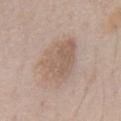This lesion was catalogued during total-body skin photography and was not selected for biopsy.
A 15 mm close-up tile from a total-body photography series done for melanoma screening.
The lesion-visualizer software estimated an average lesion color of about L≈58 a*≈15 b*≈26 (CIELAB) and about 8 CIELAB-L* units darker than the surrounding skin. The analysis additionally found a nevus-likeness score of about 10/100 and a detector confidence of about 100 out of 100 that the crop contains a lesion.
The lesion's longest dimension is about 6 mm.
The lesion is on the chest.
Imaged with white-light lighting.
A male patient aged approximately 70.